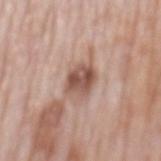{
  "biopsy_status": "not biopsied; imaged during a skin examination",
  "automated_metrics": {
    "border_irregularity_0_10": 2.0,
    "color_variation_0_10": 5.5,
    "peripheral_color_asymmetry": 2.0
  },
  "patient": {
    "sex": "male",
    "age_approx": 75
  },
  "lesion_size": {
    "long_diameter_mm_approx": 4.0
  },
  "site": "back",
  "image": {
    "source": "total-body photography crop",
    "field_of_view_mm": 15
  },
  "lighting": "white-light"
}